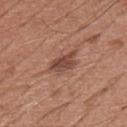Captured during whole-body skin photography for melanoma surveillance; the lesion was not biopsied.
Automated tile analysis of the lesion measured a footprint of about 6.5 mm², an eccentricity of roughly 0.85, and a symmetry-axis asymmetry near 0.3. It also reported border irregularity of about 3.5 on a 0–10 scale and a peripheral color-asymmetry measure near 1. It also reported a nevus-likeness score of about 80/100 and a detector confidence of about 100 out of 100 that the crop contains a lesion.
A close-up tile cropped from a whole-body skin photograph, about 15 mm across.
A male subject, aged approximately 65.
About 4 mm across.
The tile uses white-light illumination.
From the chest.